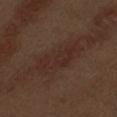Recorded during total-body skin imaging; not selected for excision or biopsy. A region of skin cropped from a whole-body photographic capture, roughly 15 mm wide. A male patient, in their 70s. Measured at roughly 6.5 mm in maximum diameter. Automated tile analysis of the lesion measured an area of roughly 14 mm² and an eccentricity of roughly 0.95. The analysis additionally found about 5 CIELAB-L* units darker than the surrounding skin and a lesion-to-skin contrast of about 5.5 (normalized; higher = more distinct). Located on the left upper arm.